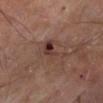Case summary:
* workup · total-body-photography surveillance lesion; no biopsy
* image · ~15 mm tile from a whole-body skin photo
* illumination · cross-polarized
* image-analysis metrics · a border-irregularity index near 5.5/10, a within-lesion color-variation index near 9.5/10, and radial color variation of about 3.5; a nevus-likeness score of about 10/100 and a detector confidence of about 100 out of 100 that the crop contains a lesion
* site · the left lower leg
* size · ~4.5 mm (longest diameter)
* subject · male, aged 53 to 57